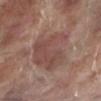Assessment:
Imaged during a routine full-body skin examination; the lesion was not biopsied and no histopathology is available.
Clinical summary:
This is a white-light tile. The lesion's longest dimension is about 6.5 mm. A 15 mm close-up extracted from a 3D total-body photography capture. A male patient about 70 years old. From the left lower leg.